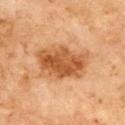notes: total-body-photography surveillance lesion; no biopsy | body site: the upper back | automated metrics: an area of roughly 19 mm², an eccentricity of roughly 0.75, and a symmetry-axis asymmetry near 0.2; a border-irregularity rating of about 3/10 and radial color variation of about 2 | lesion diameter: about 6 mm | image source: 15 mm crop, total-body photography | lighting: cross-polarized illumination | subject: male, aged 68 to 72.